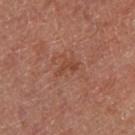This lesion was catalogued during total-body skin photography and was not selected for biopsy.
A female subject.
This is a cross-polarized tile.
The lesion is located on the right thigh.
The recorded lesion diameter is about 3 mm.
Cropped from a whole-body photographic skin survey; the tile spans about 15 mm.